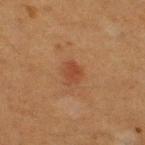About 2.5 mm across. The lesion is on the right thigh. A 15 mm close-up extracted from a 3D total-body photography capture. A female subject, approximately 50 years of age. The lesion-visualizer software estimated a mean CIELAB color near L≈37 a*≈21 b*≈29, roughly 6 lightness units darker than nearby skin, and a normalized border contrast of about 6. The software also gave border irregularity of about 2.5 on a 0–10 scale, internal color variation of about 1.5 on a 0–10 scale, and peripheral color asymmetry of about 0.5.